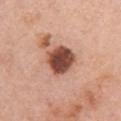The lesion was tiled from a total-body skin photograph and was not biopsied.
A close-up tile cropped from a whole-body skin photograph, about 15 mm across.
Approximately 4 mm at its widest.
A female subject, in their 60s.
The total-body-photography lesion software estimated a lesion area of about 11 mm², a shape eccentricity near 0.4, and a shape-asymmetry score of about 0.2 (0 = symmetric).
The lesion is on the right upper arm.